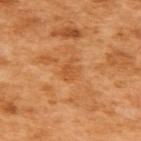Context:
The lesion is on the upper back. A female patient aged around 55. A 15 mm close-up extracted from a 3D total-body photography capture. Imaged with cross-polarized lighting. About 2.5 mm across. The lesion-visualizer software estimated a color-variation rating of about 1/10 and a peripheral color-asymmetry measure near 0.5. The analysis additionally found an automated nevus-likeness rating near 0 out of 100 and a lesion-detection confidence of about 100/100.